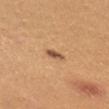biopsy status = no biopsy performed (imaged during a skin exam)
lesion size = about 2.5 mm
location = the chest
TBP lesion metrics = an area of roughly 2.5 mm², a shape eccentricity near 0.9, and two-axis asymmetry of about 0.25; a lesion color around L≈53 a*≈21 b*≈34 in CIELAB, a lesion–skin lightness drop of about 14, and a normalized border contrast of about 9.5; a within-lesion color-variation index near 0/10 and radial color variation of about 0
acquisition = 15 mm crop, total-body photography
illumination = white-light illumination
subject = female, aged approximately 25